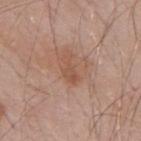Q: Was this lesion biopsied?
A: imaged on a skin check; not biopsied
Q: What did automated image analysis measure?
A: a footprint of about 4.5 mm², an outline eccentricity of about 0.9 (0 = round, 1 = elongated), and a symmetry-axis asymmetry near 0.3
Q: How large is the lesion?
A: about 3.5 mm
Q: How was the tile lit?
A: white-light
Q: How was this image acquired?
A: ~15 mm crop, total-body skin-cancer survey
Q: Who is the patient?
A: male, approximately 55 years of age
Q: What is the anatomic site?
A: the mid back